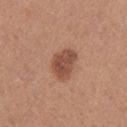Case summary:
* notes — no biopsy performed (imaged during a skin exam)
* image source — total-body-photography crop, ~15 mm field of view
* automated lesion analysis — a nevus-likeness score of about 65/100 and lesion-presence confidence of about 100/100
* location — the left thigh
* subject — female, aged 38–42
* lesion diameter — about 4 mm